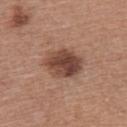Part of a total-body skin-imaging series; this lesion was reviewed on a skin check and was not flagged for biopsy.
A female patient roughly 55 years of age.
A lesion tile, about 15 mm wide, cut from a 3D total-body photograph.
Approximately 5 mm at its widest.
The tile uses white-light illumination.
The total-body-photography lesion software estimated an automated nevus-likeness rating near 85 out of 100 and a lesion-detection confidence of about 100/100.
On the upper back.